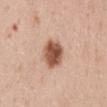Imaged during a routine full-body skin examination; the lesion was not biopsied and no histopathology is available.
Approximately 3.5 mm at its widest.
From the abdomen.
An algorithmic analysis of the crop reported an area of roughly 9.5 mm², a shape eccentricity near 0.5, and two-axis asymmetry of about 0.15. It also reported a mean CIELAB color near L≈54 a*≈23 b*≈30, a lesion–skin lightness drop of about 17, and a normalized lesion–skin contrast near 11. It also reported a border-irregularity rating of about 1.5/10 and a peripheral color-asymmetry measure near 2. The software also gave a nevus-likeness score of about 100/100 and a lesion-detection confidence of about 100/100.
A female subject, aged approximately 45.
A 15 mm crop from a total-body photograph taken for skin-cancer surveillance.
The tile uses white-light illumination.